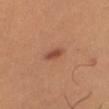Q: What is the lesion's diameter?
A: ~3 mm (longest diameter)
Q: Illumination type?
A: cross-polarized
Q: Patient demographics?
A: female, aged approximately 40
Q: Lesion location?
A: the left leg
Q: What is the imaging modality?
A: ~15 mm tile from a whole-body skin photo